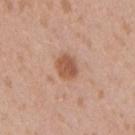<lesion>
<biopsy_status>not biopsied; imaged during a skin examination</biopsy_status>
<image>
  <source>total-body photography crop</source>
  <field_of_view_mm>15</field_of_view_mm>
</image>
<site>right upper arm</site>
<lesion_size>
  <long_diameter_mm_approx>2.5</long_diameter_mm_approx>
</lesion_size>
<lighting>white-light</lighting>
<patient>
  <sex>male</sex>
  <age_approx>35</age_approx>
</patient>
</lesion>